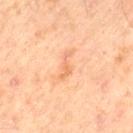Impression: Part of a total-body skin-imaging series; this lesion was reviewed on a skin check and was not flagged for biopsy. Acquisition and patient details: The lesion's longest dimension is about 3.5 mm. A male patient about 65 years old. Automated tile analysis of the lesion measured an area of roughly 3 mm² and two-axis asymmetry of about 0.55. And it measured a normalized border contrast of about 5. Imaged with cross-polarized lighting. From the left thigh. This image is a 15 mm lesion crop taken from a total-body photograph.